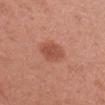The lesion was tiled from a total-body skin photograph and was not biopsied. The lesion is on the right forearm. The patient is a female about 50 years old. A 15 mm close-up extracted from a 3D total-body photography capture.